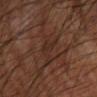No biopsy was performed on this lesion — it was imaged during a full skin examination and was not determined to be concerning. A 15 mm close-up tile from a total-body photography series done for melanoma screening. The lesion is on the leg. A male subject, in their 60s.